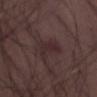Q: Was a biopsy performed?
A: total-body-photography surveillance lesion; no biopsy
Q: What are the patient's age and sex?
A: male, aged approximately 50
Q: Lesion location?
A: the left thigh
Q: What is the imaging modality?
A: total-body-photography crop, ~15 mm field of view